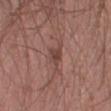Case summary:
• imaging modality · total-body-photography crop, ~15 mm field of view
• image-analysis metrics · an area of roughly 3.5 mm², an eccentricity of roughly 0.7, and a shape-asymmetry score of about 0.25 (0 = symmetric); a border-irregularity index near 2.5/10 and peripheral color asymmetry of about 1; lesion-presence confidence of about 100/100
• body site · the right lower leg
• lighting · white-light illumination
• patient · male, aged 38 to 42